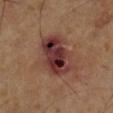The lesion was tiled from a total-body skin photograph and was not biopsied. The subject is a male in their mid-60s. A close-up tile cropped from a whole-body skin photograph, about 15 mm across. On the left leg.